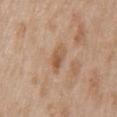notes: imaged on a skin check; not biopsied | subject: male, aged 63–67 | tile lighting: white-light illumination | imaging modality: ~15 mm tile from a whole-body skin photo | site: the chest | lesion diameter: ~3.5 mm (longest diameter).